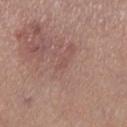Q: What is the histopathologic diagnosis?
A: a nodular basal cell carcinoma (malignant)